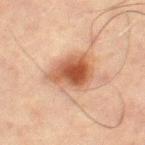Captured during whole-body skin photography for melanoma surveillance; the lesion was not biopsied.
Cropped from a whole-body photographic skin survey; the tile spans about 15 mm.
About 5 mm across.
Located on the left thigh.
A male subject, roughly 70 years of age.
The tile uses cross-polarized illumination.
The total-body-photography lesion software estimated a footprint of about 15 mm² and an eccentricity of roughly 0.65. The software also gave about 13 CIELAB-L* units darker than the surrounding skin and a normalized lesion–skin contrast near 9.5. And it measured lesion-presence confidence of about 100/100.